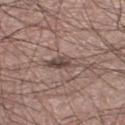Assessment:
Imaged during a routine full-body skin examination; the lesion was not biopsied and no histopathology is available.
Context:
The lesion's longest dimension is about 4 mm. A roughly 15 mm field-of-view crop from a total-body skin photograph. A male subject aged 53–57. Automated tile analysis of the lesion measured an eccentricity of roughly 0.9. The analysis additionally found a lesion color around L≈46 a*≈15 b*≈20 in CIELAB and a lesion-to-skin contrast of about 8 (normalized; higher = more distinct). The software also gave border irregularity of about 3.5 on a 0–10 scale and a within-lesion color-variation index near 6.5/10. The software also gave a detector confidence of about 70 out of 100 that the crop contains a lesion. The lesion is on the left thigh. Imaged with white-light lighting.